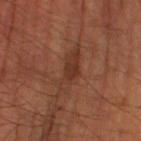notes: no biopsy performed (imaged during a skin exam)
lesion diameter: ≈6.5 mm
lighting: cross-polarized
subject: male, approximately 65 years of age
imaging modality: ~15 mm crop, total-body skin-cancer survey
site: the left leg